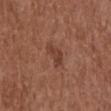The lesion was photographed on a routine skin check and not biopsied; there is no pathology result. On the upper back. A female subject, aged around 75. A 15 mm crop from a total-body photograph taken for skin-cancer surveillance. Longest diameter approximately 3.5 mm. Imaged with white-light lighting. An algorithmic analysis of the crop reported border irregularity of about 4 on a 0–10 scale and peripheral color asymmetry of about 0.5.